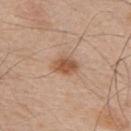Impression: This lesion was catalogued during total-body skin photography and was not selected for biopsy. Acquisition and patient details: About 3.5 mm across. Automated tile analysis of the lesion measured internal color variation of about 3.5 on a 0–10 scale. A male patient, in their 50s. A 15 mm close-up tile from a total-body photography series done for melanoma screening. Imaged with white-light lighting. On the upper back.